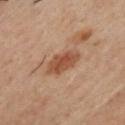Approximately 4 mm at its widest. Automated tile analysis of the lesion measured a lesion area of about 8.5 mm² and a shape-asymmetry score of about 0.15 (0 = symmetric). The analysis additionally found a border-irregularity rating of about 2/10 and a peripheral color-asymmetry measure near 1. It also reported a nevus-likeness score of about 80/100. On the chest. A 15 mm crop from a total-body photograph taken for skin-cancer surveillance. The subject is a male in their 50s. This is a cross-polarized tile.